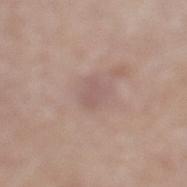Q: Was this lesion biopsied?
A: total-body-photography surveillance lesion; no biopsy
Q: Patient demographics?
A: male, about 80 years old
Q: What is the anatomic site?
A: the left lower leg
Q: Automated lesion metrics?
A: a border-irregularity index near 3/10, a color-variation rating of about 1/10, and a peripheral color-asymmetry measure near 0.5; a nevus-likeness score of about 0/100 and a detector confidence of about 100 out of 100 that the crop contains a lesion
Q: How was this image acquired?
A: ~15 mm tile from a whole-body skin photo